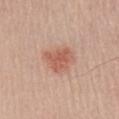Case summary:
• workup — total-body-photography surveillance lesion; no biopsy
• tile lighting — white-light
• patient — male, aged 68 to 72
• image source — 15 mm crop, total-body photography
• location — the right upper arm
• automated metrics — an eccentricity of roughly 0.55 and a shape-asymmetry score of about 0.35 (0 = symmetric); an average lesion color of about L≈57 a*≈25 b*≈30 (CIELAB), about 10 CIELAB-L* units darker than the surrounding skin, and a normalized border contrast of about 7